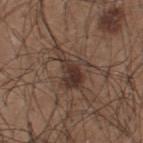biopsy_status: not biopsied; imaged during a skin examination
image:
  source: total-body photography crop
  field_of_view_mm: 15
automated_metrics:
  cielab_L: 34
  cielab_a: 15
  cielab_b: 22
  vs_skin_darker_L: 10.0
  vs_skin_contrast_norm: 9.0
lesion_size:
  long_diameter_mm_approx: 5.0
lighting: white-light
site: upper back
patient:
  sex: male
  age_approx: 25